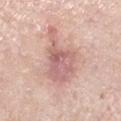notes: total-body-photography surveillance lesion; no biopsy
lesion size: about 7 mm
subject: male, aged 58 to 62
acquisition: total-body-photography crop, ~15 mm field of view
body site: the left lower leg
TBP lesion metrics: a lesion color around L≈63 a*≈22 b*≈23 in CIELAB, about 11 CIELAB-L* units darker than the surrounding skin, and a normalized border contrast of about 7; a border-irregularity rating of about 6/10, a color-variation rating of about 5.5/10, and peripheral color asymmetry of about 1.5
lighting: white-light illumination